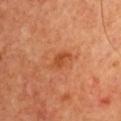| field | value |
|---|---|
| biopsy status | total-body-photography surveillance lesion; no biopsy |
| imaging modality | 15 mm crop, total-body photography |
| body site | the chest |
| subject | male, roughly 70 years of age |
| lesion size | about 3 mm |